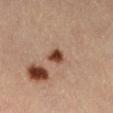Q: How was the tile lit?
A: cross-polarized illumination
Q: What is the imaging modality?
A: ~15 mm crop, total-body skin-cancer survey
Q: What are the patient's age and sex?
A: female, about 45 years old
Q: What is the anatomic site?
A: the leg